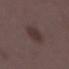follow-up = no biopsy performed (imaged during a skin exam) | patient = female, aged 33–37 | automated lesion analysis = internal color variation of about 2 on a 0–10 scale and peripheral color asymmetry of about 0.5; a nevus-likeness score of about 45/100 | illumination = white-light | location = the right lower leg | image source = total-body-photography crop, ~15 mm field of view.